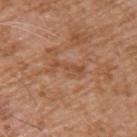| feature | finding |
|---|---|
| workup | catalogued during a skin exam; not biopsied |
| location | the left upper arm |
| lesion diameter | about 3.5 mm |
| tile lighting | white-light illumination |
| acquisition | 15 mm crop, total-body photography |
| subject | male, in their mid- to late 70s |